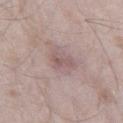Imaged during a routine full-body skin examination; the lesion was not biopsied and no histopathology is available.
A male patient aged approximately 45.
The tile uses white-light illumination.
The lesion's longest dimension is about 2.5 mm.
A region of skin cropped from a whole-body photographic capture, roughly 15 mm wide.
On the right thigh.